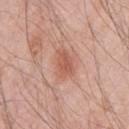This lesion was catalogued during total-body skin photography and was not selected for biopsy. The lesion-visualizer software estimated two-axis asymmetry of about 0.3. It also reported a mean CIELAB color near L≈57 a*≈25 b*≈30 and roughly 9 lightness units darker than nearby skin. And it measured a border-irregularity index near 3/10 and radial color variation of about 0.5. It also reported a classifier nevus-likeness of about 65/100. The lesion is on the left upper arm. The subject is a male approximately 45 years of age. A roughly 15 mm field-of-view crop from a total-body skin photograph. The lesion's longest dimension is about 3.5 mm.